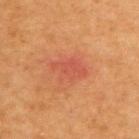Clinical impression: This lesion was catalogued during total-body skin photography and was not selected for biopsy. Context: A male patient aged 63 to 67. The lesion is on the upper back. Measured at roughly 4 mm in maximum diameter. A region of skin cropped from a whole-body photographic capture, roughly 15 mm wide. Captured under cross-polarized illumination.